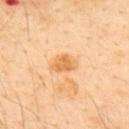| field | value |
|---|---|
| biopsy status | imaged on a skin check; not biopsied |
| patient | male, aged 48 to 52 |
| image source | ~15 mm crop, total-body skin-cancer survey |
| automated lesion analysis | roughly 10 lightness units darker than nearby skin and a normalized lesion–skin contrast near 7.5 |
| body site | the upper back |